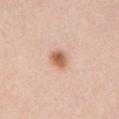Impression:
No biopsy was performed on this lesion — it was imaged during a full skin examination and was not determined to be concerning.
Acquisition and patient details:
A female patient, roughly 50 years of age. Located on the abdomen. Cropped from a total-body skin-imaging series; the visible field is about 15 mm.